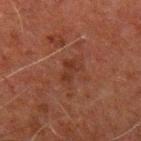No biopsy was performed on this lesion — it was imaged during a full skin examination and was not determined to be concerning. A roughly 15 mm field-of-view crop from a total-body skin photograph. The subject is a male aged approximately 60. From the left upper arm.